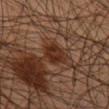No biopsy was performed on this lesion — it was imaged during a full skin examination and was not determined to be concerning.
The tile uses cross-polarized illumination.
Cropped from a whole-body photographic skin survey; the tile spans about 15 mm.
From the right lower leg.
A male patient, roughly 45 years of age.
The lesion's longest dimension is about 3 mm.